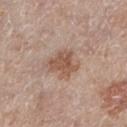| feature | finding |
|---|---|
| biopsy status | imaged on a skin check; not biopsied |